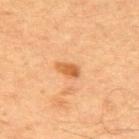Notes:
• biopsy status · imaged on a skin check; not biopsied
• location · the back
• image-analysis metrics · a border-irregularity index near 2.5/10
• size · about 3 mm
• image source · ~15 mm crop, total-body skin-cancer survey
• subject · male, aged around 65
• tile lighting · cross-polarized illumination